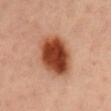Imaged during a routine full-body skin examination; the lesion was not biopsied and no histopathology is available. Imaged with cross-polarized lighting. The subject is a male aged around 55. The recorded lesion diameter is about 6 mm. A 15 mm close-up tile from a total-body photography series done for melanoma screening. On the mid back.